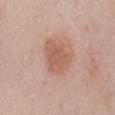Clinical summary:
A 15 mm close-up tile from a total-body photography series done for melanoma screening. The lesion is located on the mid back. A male subject, in their mid-50s.